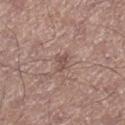Recorded during total-body skin imaging; not selected for excision or biopsy.
A 15 mm close-up tile from a total-body photography series done for melanoma screening.
Located on the right lower leg.
This is a white-light tile.
A male patient aged 68 to 72.
Measured at roughly 2.5 mm in maximum diameter.